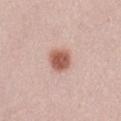Assessment: The lesion was tiled from a total-body skin photograph and was not biopsied. Context: Located on the front of the torso. The recorded lesion diameter is about 3 mm. This is a white-light tile. Cropped from a total-body skin-imaging series; the visible field is about 15 mm. A female patient, aged 23–27.